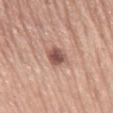workup: imaged on a skin check; not biopsied
image: ~15 mm crop, total-body skin-cancer survey
site: the mid back
TBP lesion metrics: a mean CIELAB color near L≈53 a*≈22 b*≈25, a lesion–skin lightness drop of about 14, and a lesion-to-skin contrast of about 9.5 (normalized; higher = more distinct); border irregularity of about 2 on a 0–10 scale and a peripheral color-asymmetry measure near 1; a nevus-likeness score of about 85/100 and lesion-presence confidence of about 100/100
diameter: ~2.5 mm (longest diameter)
illumination: white-light illumination
subject: male, aged 63 to 67